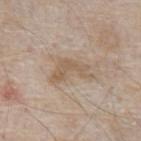Context:
A region of skin cropped from a whole-body photographic capture, roughly 15 mm wide. Measured at roughly 5 mm in maximum diameter. A male subject approximately 65 years of age. On the abdomen. The tile uses white-light illumination.